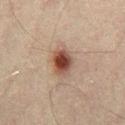| key | value |
|---|---|
| biopsy status | catalogued during a skin exam; not biopsied |
| image source | ~15 mm crop, total-body skin-cancer survey |
| patient | male, aged 38 to 42 |
| body site | the right thigh |